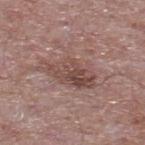workup: catalogued during a skin exam; not biopsied | location: the upper back | subject: male, roughly 65 years of age | illumination: white-light illumination | size: ~9 mm (longest diameter) | image: ~15 mm crop, total-body skin-cancer survey.